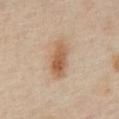This lesion was catalogued during total-body skin photography and was not selected for biopsy.